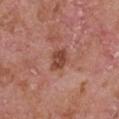Q: Was this lesion biopsied?
A: catalogued during a skin exam; not biopsied
Q: How large is the lesion?
A: ≈3 mm
Q: Where on the body is the lesion?
A: the front of the torso
Q: Illumination type?
A: white-light
Q: What are the patient's age and sex?
A: male, roughly 65 years of age
Q: How was this image acquired?
A: ~15 mm crop, total-body skin-cancer survey
Q: Automated lesion metrics?
A: an eccentricity of roughly 0.65 and two-axis asymmetry of about 0.2; a border-irregularity rating of about 2/10, a color-variation rating of about 2.5/10, and peripheral color asymmetry of about 1